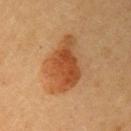biopsy status: no biopsy performed (imaged during a skin exam) | imaging modality: 15 mm crop, total-body photography | size: ~6.5 mm (longest diameter) | image-analysis metrics: lesion-presence confidence of about 100/100 | patient: female, about 60 years old | body site: the right upper arm.